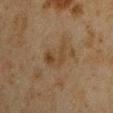<lesion>
  <lesion_size>
    <long_diameter_mm_approx>4.0</long_diameter_mm_approx>
  </lesion_size>
  <image>
    <source>total-body photography crop</source>
    <field_of_view_mm>15</field_of_view_mm>
  </image>
  <patient>
    <sex>male</sex>
    <age_approx>45</age_approx>
  </patient>
  <site>right upper arm</site>
  <lighting>cross-polarized</lighting>
</lesion>